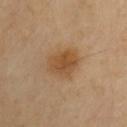* follow-up: imaged on a skin check; not biopsied
* image-analysis metrics: an average lesion color of about L≈51 a*≈19 b*≈37 (CIELAB), a lesion–skin lightness drop of about 9, and a lesion-to-skin contrast of about 7.5 (normalized; higher = more distinct)
* lesion size: ≈4.5 mm
* location: the arm
* illumination: cross-polarized illumination
* image: total-body-photography crop, ~15 mm field of view